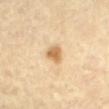Clinical impression:
Part of a total-body skin-imaging series; this lesion was reviewed on a skin check and was not flagged for biopsy.
Acquisition and patient details:
A region of skin cropped from a whole-body photographic capture, roughly 15 mm wide. The recorded lesion diameter is about 2.5 mm. A female patient, about 65 years old. The lesion is on the mid back.